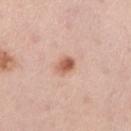Impression:
This lesion was catalogued during total-body skin photography and was not selected for biopsy.
Clinical summary:
A lesion tile, about 15 mm wide, cut from a 3D total-body photograph. From the right lower leg. Longest diameter approximately 2.5 mm. The patient is a female approximately 45 years of age. This is a white-light tile.